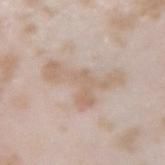notes: total-body-photography surveillance lesion; no biopsy.